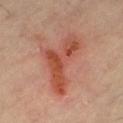{"biopsy_status": "not biopsied; imaged during a skin examination", "patient": {"sex": "male", "age_approx": 55}, "lighting": "cross-polarized", "site": "leg", "image": {"source": "total-body photography crop", "field_of_view_mm": 15}}